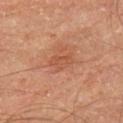Assessment: Captured during whole-body skin photography for melanoma surveillance; the lesion was not biopsied. Clinical summary: A male subject about 65 years old. The tile uses cross-polarized illumination. From the upper back. The lesion-visualizer software estimated a symmetry-axis asymmetry near 0.35. And it measured lesion-presence confidence of about 100/100. The lesion's longest dimension is about 3 mm. A roughly 15 mm field-of-view crop from a total-body skin photograph.